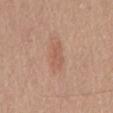<record>
<biopsy_status>not biopsied; imaged during a skin examination</biopsy_status>
<image>
  <source>total-body photography crop</source>
  <field_of_view_mm>15</field_of_view_mm>
</image>
<lesion_size>
  <long_diameter_mm_approx>3.5</long_diameter_mm_approx>
</lesion_size>
<patient>
  <sex>male</sex>
  <age_approx>65</age_approx>
</patient>
<site>back</site>
</record>